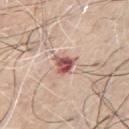Findings:
– image source · 15 mm crop, total-body photography
– automated lesion analysis · an area of roughly 4.5 mm², an outline eccentricity of about 0.3 (0 = round, 1 = elongated), and two-axis asymmetry of about 0.35; an average lesion color of about L≈54 a*≈25 b*≈23 (CIELAB), about 17 CIELAB-L* units darker than the surrounding skin, and a lesion-to-skin contrast of about 11 (normalized; higher = more distinct)
– lesion size · ≈2.5 mm
– patient · male, aged approximately 70
– location · the chest